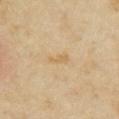Part of a total-body skin-imaging series; this lesion was reviewed on a skin check and was not flagged for biopsy. A 15 mm close-up extracted from a 3D total-body photography capture. Longest diameter approximately 3 mm. A female subject, roughly 35 years of age. The lesion-visualizer software estimated an area of roughly 3 mm² and an outline eccentricity of about 0.9 (0 = round, 1 = elongated). The analysis additionally found a lesion–skin lightness drop of about 6 and a normalized lesion–skin contrast near 5. It also reported a border-irregularity index near 4/10, internal color variation of about 0.5 on a 0–10 scale, and radial color variation of about 0. The software also gave an automated nevus-likeness rating near 0 out of 100 and a lesion-detection confidence of about 100/100. The lesion is located on the right upper arm.